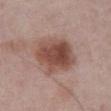Q: Was a biopsy performed?
A: no biopsy performed (imaged during a skin exam)
Q: How was this image acquired?
A: total-body-photography crop, ~15 mm field of view
Q: Illumination type?
A: white-light illumination
Q: How large is the lesion?
A: about 5.5 mm
Q: Lesion location?
A: the abdomen
Q: Who is the patient?
A: male, in their mid- to late 70s